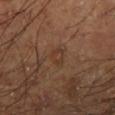The tile uses cross-polarized illumination. The patient is a male in their mid- to late 60s. An algorithmic analysis of the crop reported a mean CIELAB color near L≈36 a*≈19 b*≈28, about 5 CIELAB-L* units darker than the surrounding skin, and a lesion-to-skin contrast of about 4.5 (normalized; higher = more distinct). The analysis additionally found border irregularity of about 2.5 on a 0–10 scale, internal color variation of about 2 on a 0–10 scale, and radial color variation of about 0.5. And it measured a nevus-likeness score of about 0/100 and a detector confidence of about 100 out of 100 that the crop contains a lesion. The recorded lesion diameter is about 3 mm. On the left lower leg. A 15 mm close-up extracted from a 3D total-body photography capture.